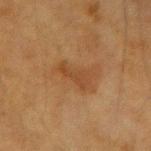Context: A region of skin cropped from a whole-body photographic capture, roughly 15 mm wide. About 3.5 mm across. Imaged with cross-polarized lighting. Automated tile analysis of the lesion measured a footprint of about 4 mm² and a shape eccentricity near 0.95. The analysis additionally found a mean CIELAB color near L≈36 a*≈19 b*≈31 and roughly 6 lightness units darker than nearby skin. The software also gave a classifier nevus-likeness of about 0/100 and a detector confidence of about 100 out of 100 that the crop contains a lesion. From the left forearm. A female subject, aged 53 to 57.